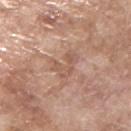anatomic site: the upper back | lesion size: ~3 mm (longest diameter) | illumination: white-light | patient: male, in their mid-60s | automated metrics: a border-irregularity index near 7/10, a within-lesion color-variation index near 2/10, and radial color variation of about 0.5; lesion-presence confidence of about 95/100 | imaging modality: total-body-photography crop, ~15 mm field of view.